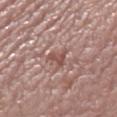Captured during whole-body skin photography for melanoma surveillance; the lesion was not biopsied. The tile uses white-light illumination. The recorded lesion diameter is about 2.5 mm. The lesion is on the right thigh. A female subject approximately 65 years of age. A close-up tile cropped from a whole-body skin photograph, about 15 mm across.